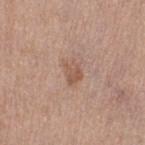The lesion was photographed on a routine skin check and not biopsied; there is no pathology result.
Automated image analysis of the tile measured an area of roughly 4.5 mm² and an eccentricity of roughly 0.7. The analysis additionally found a lesion color around L≈55 a*≈20 b*≈28 in CIELAB and about 8 CIELAB-L* units darker than the surrounding skin. It also reported a nevus-likeness score of about 5/100 and a detector confidence of about 100 out of 100 that the crop contains a lesion.
On the left thigh.
Imaged with white-light lighting.
A female patient in their 40s.
The lesion's longest dimension is about 3 mm.
A lesion tile, about 15 mm wide, cut from a 3D total-body photograph.